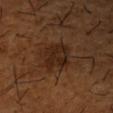Q: Lesion location?
A: the right forearm
Q: What lighting was used for the tile?
A: cross-polarized
Q: How was this image acquired?
A: ~15 mm tile from a whole-body skin photo
Q: What is the lesion's diameter?
A: ~4 mm (longest diameter)
Q: Patient demographics?
A: male, aged approximately 65